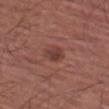The lesion was tiled from a total-body skin photograph and was not biopsied. A male patient, aged approximately 65. The lesion is located on the left thigh. Imaged with white-light lighting. A roughly 15 mm field-of-view crop from a total-body skin photograph.